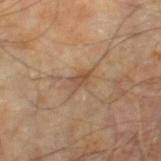Clinical summary: A roughly 15 mm field-of-view crop from a total-body skin photograph. The subject is a male aged 58 to 62. The lesion is on the leg. Approximately 3 mm at its widest.